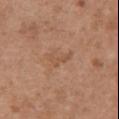Image and clinical context:
An algorithmic analysis of the crop reported an outline eccentricity of about 0.9 (0 = round, 1 = elongated) and a shape-asymmetry score of about 0.7 (0 = symmetric). The analysis additionally found a mean CIELAB color near L≈52 a*≈21 b*≈32, roughly 6 lightness units darker than nearby skin, and a lesion-to-skin contrast of about 5 (normalized; higher = more distinct). And it measured a border-irregularity rating of about 7.5/10, a within-lesion color-variation index near 0/10, and peripheral color asymmetry of about 0. Imaged with white-light lighting. A region of skin cropped from a whole-body photographic capture, roughly 15 mm wide. The subject is a female roughly 65 years of age. The lesion is on the chest. The recorded lesion diameter is about 3 mm.